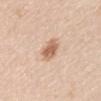{"biopsy_status": "not biopsied; imaged during a skin examination", "site": "right upper arm", "automated_metrics": {"area_mm2_approx": 6.0, "eccentricity": 0.7, "shape_asymmetry": 0.2, "border_irregularity_0_10": 2.0, "color_variation_0_10": 3.5, "peripheral_color_asymmetry": 1.0}, "lighting": "white-light", "lesion_size": {"long_diameter_mm_approx": 3.0}, "patient": {"sex": "male", "age_approx": 45}, "image": {"source": "total-body photography crop", "field_of_view_mm": 15}}